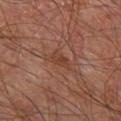This image is a 15 mm lesion crop taken from a total-body photograph.
A male patient, approximately 60 years of age.
Measured at roughly 2.5 mm in maximum diameter.
The lesion is on the right leg.
This is a cross-polarized tile.